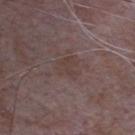Assessment:
This lesion was catalogued during total-body skin photography and was not selected for biopsy.
Background:
Automated image analysis of the tile measured border irregularity of about 5 on a 0–10 scale, internal color variation of about 1.5 on a 0–10 scale, and a peripheral color-asymmetry measure near 0.5. A male subject aged 63–67. The lesion is located on the chest. This is a white-light tile. A roughly 15 mm field-of-view crop from a total-body skin photograph.